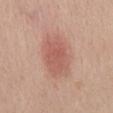Impression: The lesion was photographed on a routine skin check and not biopsied; there is no pathology result. Context: A female patient approximately 50 years of age. Approximately 5.5 mm at its widest. The lesion is located on the mid back. A lesion tile, about 15 mm wide, cut from a 3D total-body photograph.